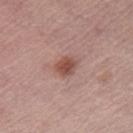| field | value |
|---|---|
| follow-up | total-body-photography surveillance lesion; no biopsy |
| subject | female, in their mid-60s |
| lesion size | about 2.5 mm |
| acquisition | total-body-photography crop, ~15 mm field of view |
| location | the right thigh |